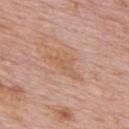The lesion was tiled from a total-body skin photograph and was not biopsied. Located on the upper back. About 5 mm across. Captured under white-light illumination. Cropped from a total-body skin-imaging series; the visible field is about 15 mm. The patient is a male aged around 75.